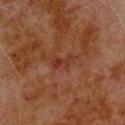| key | value |
|---|---|
| follow-up | total-body-photography surveillance lesion; no biopsy |
| acquisition | ~15 mm crop, total-body skin-cancer survey |
| patient | male, aged 78 to 82 |
| diameter | about 3 mm |
| tile lighting | cross-polarized illumination |
| body site | the upper back |